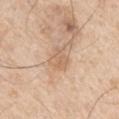Clinical impression:
Imaged during a routine full-body skin examination; the lesion was not biopsied and no histopathology is available.
Background:
Captured under white-light illumination. This image is a 15 mm lesion crop taken from a total-body photograph. The lesion's longest dimension is about 3 mm. A male patient in their mid-50s. An algorithmic analysis of the crop reported an area of roughly 5 mm², an outline eccentricity of about 0.6 (0 = round, 1 = elongated), and a shape-asymmetry score of about 0.3 (0 = symmetric). And it measured a mean CIELAB color near L≈63 a*≈18 b*≈33, a lesion–skin lightness drop of about 7, and a lesion-to-skin contrast of about 5 (normalized; higher = more distinct). The lesion is located on the left upper arm.